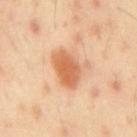notes: no biopsy performed (imaged during a skin exam)
imaging modality: ~15 mm crop, total-body skin-cancer survey
patient: male, aged approximately 45
diameter: ~4.5 mm (longest diameter)
anatomic site: the mid back